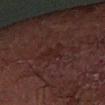Assessment:
The lesion was tiled from a total-body skin photograph and was not biopsied.
Image and clinical context:
The subject is a male in their 70s. Approximately 3 mm at its widest. Automated image analysis of the tile measured a footprint of about 4.5 mm² and a symmetry-axis asymmetry near 0.4. The analysis additionally found an average lesion color of about L≈17 a*≈16 b*≈16 (CIELAB), about 3 CIELAB-L* units darker than the surrounding skin, and a normalized border contrast of about 4.5. It also reported border irregularity of about 5 on a 0–10 scale, a within-lesion color-variation index near 1/10, and a peripheral color-asymmetry measure near 0.5. A lesion tile, about 15 mm wide, cut from a 3D total-body photograph. The lesion is located on the right forearm. This is a cross-polarized tile.